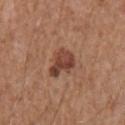Findings:
* patient: male, aged 63 to 67
* image: total-body-photography crop, ~15 mm field of view
* site: the chest